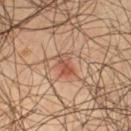• workup · total-body-photography surveillance lesion; no biopsy
• imaging modality · total-body-photography crop, ~15 mm field of view
• patient · male, aged approximately 55
• location · the left thigh
• image-analysis metrics · a shape eccentricity near 0.75